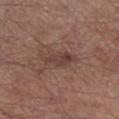Clinical impression: Recorded during total-body skin imaging; not selected for excision or biopsy. Clinical summary: A male subject, aged 53 to 57. A region of skin cropped from a whole-body photographic capture, roughly 15 mm wide. From the right lower leg. This is a white-light tile. The recorded lesion diameter is about 4.5 mm.